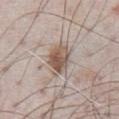Background: Imaged with white-light lighting. A male subject, aged around 70. A lesion tile, about 15 mm wide, cut from a 3D total-body photograph. On the front of the torso. The recorded lesion diameter is about 4.5 mm.